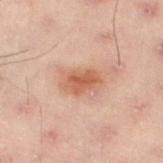<tbp_lesion>
<image>
  <source>total-body photography crop</source>
  <field_of_view_mm>15</field_of_view_mm>
</image>
<patient>
  <sex>male</sex>
  <age_approx>50</age_approx>
</patient>
<site>left lower leg</site>
<automated_metrics>
  <eccentricity>0.8</eccentricity>
  <shape_asymmetry>0.2</shape_asymmetry>
  <border_irregularity_0_10>2.5</border_irregularity_0_10>
  <color_variation_0_10>3.0</color_variation_0_10>
  <peripheral_color_asymmetry>1.0</peripheral_color_asymmetry>
  <nevus_likeness_0_100>85</nevus_likeness_0_100>
  <lesion_detection_confidence_0_100>100</lesion_detection_confidence_0_100>
</automated_metrics>
<lesion_size>
  <long_diameter_mm_approx>4.0</long_diameter_mm_approx>
</lesion_size>
<lighting>cross-polarized</lighting>
</tbp_lesion>